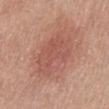Q: How was this image acquired?
A: ~15 mm tile from a whole-body skin photo
Q: Where on the body is the lesion?
A: the back
Q: Illumination type?
A: white-light
Q: How large is the lesion?
A: ≈6 mm
Q: Who is the patient?
A: male, roughly 55 years of age